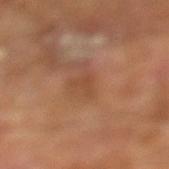Assessment:
No biopsy was performed on this lesion — it was imaged during a full skin examination and was not determined to be concerning.
Acquisition and patient details:
A male subject, aged approximately 70. On the left lower leg. Cropped from a whole-body photographic skin survey; the tile spans about 15 mm.